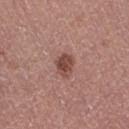The lesion was photographed on a routine skin check and not biopsied; there is no pathology result. Imaged with white-light lighting. A female subject, approximately 50 years of age. A region of skin cropped from a whole-body photographic capture, roughly 15 mm wide. The lesion is on the leg. Approximately 2.5 mm at its widest. An algorithmic analysis of the crop reported a footprint of about 5 mm², an eccentricity of roughly 0.55, and a symmetry-axis asymmetry near 0.2. It also reported a nevus-likeness score of about 90/100 and lesion-presence confidence of about 100/100.